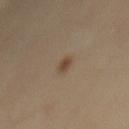Part of a total-body skin-imaging series; this lesion was reviewed on a skin check and was not flagged for biopsy. Imaged with cross-polarized lighting. Longest diameter approximately 2 mm. From the back. Cropped from a total-body skin-imaging series; the visible field is about 15 mm. A male patient roughly 55 years of age. Automated image analysis of the tile measured an outline eccentricity of about 0.8 (0 = round, 1 = elongated). And it measured an average lesion color of about L≈44 a*≈15 b*≈28 (CIELAB) and roughly 9 lightness units darker than nearby skin. The software also gave a border-irregularity index near 2.5/10, a color-variation rating of about 2.5/10, and a peripheral color-asymmetry measure near 1. And it measured a nevus-likeness score of about 90/100.